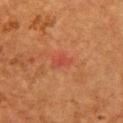notes: imaged on a skin check; not biopsied
acquisition: ~15 mm crop, total-body skin-cancer survey
size: about 2.5 mm
body site: the front of the torso
patient: female, approximately 50 years of age
automated metrics: a lesion area of about 3.5 mm², a shape eccentricity near 0.8, and a symmetry-axis asymmetry near 0.3; a lesion color around L≈40 a*≈29 b*≈29 in CIELAB and a normalized lesion–skin contrast near 5; a border-irregularity rating of about 2.5/10 and a color-variation rating of about 1.5/10